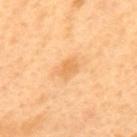Q: Was a biopsy performed?
A: total-body-photography surveillance lesion; no biopsy
Q: Lesion location?
A: the mid back
Q: How large is the lesion?
A: about 2.5 mm
Q: What did automated image analysis measure?
A: a footprint of about 4 mm², a shape eccentricity near 0.7, and a shape-asymmetry score of about 0.25 (0 = symmetric)
Q: Who is the patient?
A: male, in their 50s
Q: What kind of image is this?
A: 15 mm crop, total-body photography
Q: What lighting was used for the tile?
A: cross-polarized